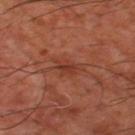Clinical impression: This lesion was catalogued during total-body skin photography and was not selected for biopsy. Image and clinical context: From the leg. Imaged with cross-polarized lighting. Approximately 3 mm at its widest. A close-up tile cropped from a whole-body skin photograph, about 15 mm across. The subject is in their mid- to late 60s.